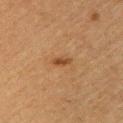This lesion was catalogued during total-body skin photography and was not selected for biopsy.
A 15 mm close-up extracted from a 3D total-body photography capture.
Located on the front of the torso.
The tile uses cross-polarized illumination.
A male subject aged 63 to 67.
Approximately 2.5 mm at its widest.